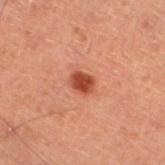Imaged during a routine full-body skin examination; the lesion was not biopsied and no histopathology is available.